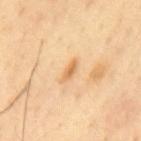Findings:
• follow-up — catalogued during a skin exam; not biopsied
• patient — male, about 40 years old
• lighting — cross-polarized illumination
• diameter — about 2.5 mm
• imaging modality — 15 mm crop, total-body photography
• body site — the back